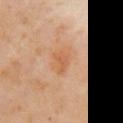Part of a total-body skin-imaging series; this lesion was reviewed on a skin check and was not flagged for biopsy.